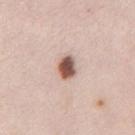Clinical impression:
Part of a total-body skin-imaging series; this lesion was reviewed on a skin check and was not flagged for biopsy.
Clinical summary:
The lesion is on the chest. A female subject, in their 40s. A close-up tile cropped from a whole-body skin photograph, about 15 mm across.